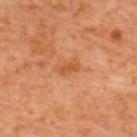tile lighting: cross-polarized | imaging modality: total-body-photography crop, ~15 mm field of view | automated metrics: a footprint of about 3.5 mm², an eccentricity of roughly 0.9, and a shape-asymmetry score of about 0.3 (0 = symmetric); an average lesion color of about L≈54 a*≈28 b*≈42 (CIELAB), roughly 7 lightness units darker than nearby skin, and a lesion-to-skin contrast of about 6 (normalized; higher = more distinct); a border-irregularity index near 2.5/10, a within-lesion color-variation index near 1.5/10, and peripheral color asymmetry of about 0.5 | anatomic site: the upper back | patient: aged approximately 65 | lesion diameter: about 3 mm.